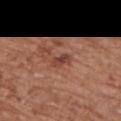Imaged with white-light lighting.
Automated image analysis of the tile measured a lesion area of about 3 mm², an outline eccentricity of about 0.85 (0 = round, 1 = elongated), and a shape-asymmetry score of about 0.25 (0 = symmetric). The software also gave a mean CIELAB color near L≈43 a*≈24 b*≈27, a lesion–skin lightness drop of about 9, and a normalized lesion–skin contrast near 7. The software also gave a nevus-likeness score of about 0/100 and a lesion-detection confidence of about 100/100.
The patient is a female aged approximately 75.
On the back.
A close-up tile cropped from a whole-body skin photograph, about 15 mm across.
The lesion's longest dimension is about 2.5 mm.